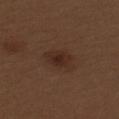Impression: This lesion was catalogued during total-body skin photography and was not selected for biopsy. Image and clinical context: The recorded lesion diameter is about 4 mm. The lesion is on the left upper arm. This is a white-light tile. The patient is a female aged 28 to 32. A lesion tile, about 15 mm wide, cut from a 3D total-body photograph.